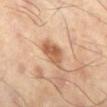– image source · ~15 mm crop, total-body skin-cancer survey
– body site · the right lower leg
– automated lesion analysis · a normalized border contrast of about 8; a border-irregularity index near 2.5/10, internal color variation of about 4.5 on a 0–10 scale, and peripheral color asymmetry of about 1.5
– lesion diameter · ≈4 mm
– patient · male, roughly 65 years of age
– illumination · cross-polarized illumination